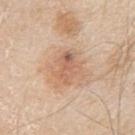No biopsy was performed on this lesion — it was imaged during a full skin examination and was not determined to be concerning.
Imaged with white-light lighting.
A male subject about 80 years old.
About 4 mm across.
A lesion tile, about 15 mm wide, cut from a 3D total-body photograph.
Located on the arm.
Automated tile analysis of the lesion measured a mean CIELAB color near L≈62 a*≈20 b*≈32, roughly 9 lightness units darker than nearby skin, and a normalized lesion–skin contrast near 6. And it measured border irregularity of about 3.5 on a 0–10 scale, a within-lesion color-variation index near 6.5/10, and radial color variation of about 2.